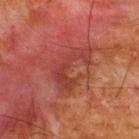Q: Is there a histopathology result?
A: imaged on a skin check; not biopsied
Q: What are the patient's age and sex?
A: male, approximately 65 years of age
Q: What is the imaging modality?
A: ~15 mm crop, total-body skin-cancer survey
Q: How was the tile lit?
A: cross-polarized
Q: What is the lesion's diameter?
A: ≈6 mm
Q: Lesion location?
A: the upper back